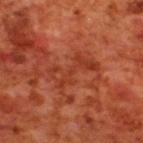biopsy status — no biopsy performed (imaged during a skin exam) | diameter — ~5 mm (longest diameter) | automated metrics — a footprint of about 9 mm², an outline eccentricity of about 0.9 (0 = round, 1 = elongated), and a shape-asymmetry score of about 0.5 (0 = symmetric); a color-variation rating of about 3.5/10 and peripheral color asymmetry of about 1; a detector confidence of about 90 out of 100 that the crop contains a lesion | location — the upper back | image — ~15 mm crop, total-body skin-cancer survey | subject — male, approximately 70 years of age.